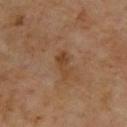biopsy status = total-body-photography surveillance lesion; no biopsy
subject = male, approximately 70 years of age
anatomic site = the upper back
acquisition = 15 mm crop, total-body photography
size = about 3 mm
tile lighting = cross-polarized illumination